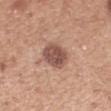Recorded during total-body skin imaging; not selected for excision or biopsy.